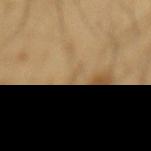<lesion>
  <patient>
    <sex>male</sex>
    <age_approx>65</age_approx>
  </patient>
  <image>
    <source>total-body photography crop</source>
    <field_of_view_mm>15</field_of_view_mm>
  </image>
  <site>mid back</site>
</lesion>